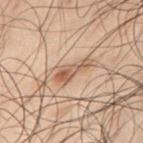Case summary:
– workup: total-body-photography surveillance lesion; no biopsy
– automated lesion analysis: a shape-asymmetry score of about 0.45 (0 = symmetric); a border-irregularity index near 5.5/10, a color-variation rating of about 3/10, and a peripheral color-asymmetry measure near 0.5
– image: ~15 mm crop, total-body skin-cancer survey
– body site: the right thigh
– subject: male, aged around 50
– tile lighting: cross-polarized illumination
– lesion size: about 4.5 mm